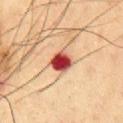notes: catalogued during a skin exam; not biopsied | subject: male, roughly 60 years of age | lesion diameter: about 3 mm | body site: the chest | imaging modality: ~15 mm tile from a whole-body skin photo | automated lesion analysis: an outline eccentricity of about 0.45 (0 = round, 1 = elongated) and a symmetry-axis asymmetry near 0.2.